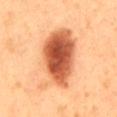Impression:
Imaged during a routine full-body skin examination; the lesion was not biopsied and no histopathology is available.
Acquisition and patient details:
The tile uses cross-polarized illumination. The lesion-visualizer software estimated a lesion area of about 33 mm² and two-axis asymmetry of about 0.2. The software also gave a mean CIELAB color near L≈62 a*≈30 b*≈41, roughly 19 lightness units darker than nearby skin, and a normalized lesion–skin contrast near 10.5. It also reported an automated nevus-likeness rating near 100 out of 100. Longest diameter approximately 9 mm. The lesion is located on the mid back. A 15 mm close-up tile from a total-body photography series done for melanoma screening. The patient is a male aged around 50.